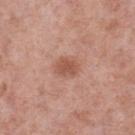The lesion is located on the left lower leg. Automated tile analysis of the lesion measured a shape eccentricity near 0.6 and a shape-asymmetry score of about 0.2 (0 = symmetric). The software also gave a lesion color around L≈54 a*≈24 b*≈29 in CIELAB, a lesion–skin lightness drop of about 9, and a lesion-to-skin contrast of about 6.5 (normalized; higher = more distinct). The software also gave a border-irregularity rating of about 2/10, a within-lesion color-variation index near 2/10, and peripheral color asymmetry of about 0.5. This is a white-light tile. A roughly 15 mm field-of-view crop from a total-body skin photograph. A male patient, aged 53 to 57.